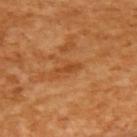| key | value |
|---|---|
| follow-up | no biopsy performed (imaged during a skin exam) |
| site | the upper back |
| acquisition | ~15 mm tile from a whole-body skin photo |
| TBP lesion metrics | an average lesion color of about L≈48 a*≈28 b*≈43 (CIELAB), a lesion–skin lightness drop of about 9, and a normalized lesion–skin contrast near 6.5; a border-irregularity index near 4/10, a within-lesion color-variation index near 0/10, and a peripheral color-asymmetry measure near 0; a classifier nevus-likeness of about 0/100 and a lesion-detection confidence of about 100/100 |
| lesion diameter | ~3 mm (longest diameter) |
| patient | female, in their mid- to late 50s |
| illumination | cross-polarized |